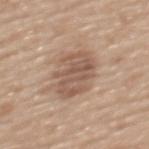The lesion was photographed on a routine skin check and not biopsied; there is no pathology result. A close-up tile cropped from a whole-body skin photograph, about 15 mm across. An algorithmic analysis of the crop reported a shape eccentricity near 0.65 and a shape-asymmetry score of about 0.2 (0 = symmetric). It also reported a normalized lesion–skin contrast near 7. The analysis additionally found border irregularity of about 3.5 on a 0–10 scale and radial color variation of about 1.5. A female patient, aged around 70. The tile uses white-light illumination. Measured at roughly 5.5 mm in maximum diameter. From the upper back.